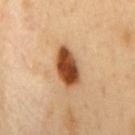Clinical impression: Part of a total-body skin-imaging series; this lesion was reviewed on a skin check and was not flagged for biopsy. Acquisition and patient details: The lesion is on the mid back. A male patient in their 50s. A 15 mm close-up tile from a total-body photography series done for melanoma screening. This is a cross-polarized tile. The recorded lesion diameter is about 5 mm.